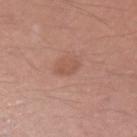{"biopsy_status": "not biopsied; imaged during a skin examination", "image": {"source": "total-body photography crop", "field_of_view_mm": 15}, "lighting": "white-light", "site": "left upper arm", "patient": {"sex": "male", "age_approx": 35}, "lesion_size": {"long_diameter_mm_approx": 2.5}, "automated_metrics": {"border_irregularity_0_10": 4.5, "color_variation_0_10": 0.0, "peripheral_color_asymmetry": 0.0, "nevus_likeness_0_100": 25, "lesion_detection_confidence_0_100": 100}}